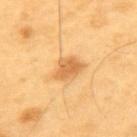Part of a total-body skin-imaging series; this lesion was reviewed on a skin check and was not flagged for biopsy.
Imaged with cross-polarized lighting.
Located on the upper back.
An algorithmic analysis of the crop reported a mean CIELAB color near L≈65 a*≈23 b*≈46 and about 12 CIELAB-L* units darker than the surrounding skin.
Measured at roughly 3.5 mm in maximum diameter.
A 15 mm close-up tile from a total-body photography series done for melanoma screening.
A male patient, about 60 years old.